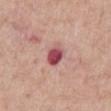  biopsy_status: not biopsied; imaged during a skin examination
  site: chest
  image:
    source: total-body photography crop
    field_of_view_mm: 15
  patient:
    sex: male
    age_approx: 60